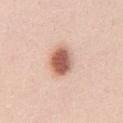<record>
  <biopsy_status>not biopsied; imaged during a skin examination</biopsy_status>
  <lesion_size>
    <long_diameter_mm_approx>4.0</long_diameter_mm_approx>
  </lesion_size>
  <image>
    <source>total-body photography crop</source>
    <field_of_view_mm>15</field_of_view_mm>
  </image>
  <patient>
    <sex>female</sex>
    <age_approx>20</age_approx>
  </patient>
  <site>front of the torso</site>
</record>